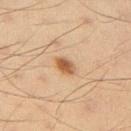This lesion was catalogued during total-body skin photography and was not selected for biopsy. A lesion tile, about 15 mm wide, cut from a 3D total-body photograph. A male patient, in their mid-30s. From the right upper arm.